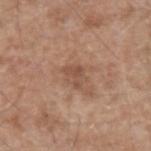biopsy status: imaged on a skin check; not biopsied | site: the left forearm | imaging modality: 15 mm crop, total-body photography | patient: male, approximately 60 years of age | automated lesion analysis: a lesion area of about 4.5 mm², an outline eccentricity of about 0.65 (0 = round, 1 = elongated), and two-axis asymmetry of about 0.3; a within-lesion color-variation index near 3/10 and radial color variation of about 1; a lesion-detection confidence of about 100/100.